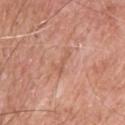The lesion was tiled from a total-body skin photograph and was not biopsied.
The tile uses white-light illumination.
A male patient, approximately 60 years of age.
About 3 mm across.
The lesion is located on the upper back.
A 15 mm close-up tile from a total-body photography series done for melanoma screening.
Automated tile analysis of the lesion measured a footprint of about 2.5 mm², a shape eccentricity near 0.95, and a shape-asymmetry score of about 0.4 (0 = symmetric). The software also gave a nevus-likeness score of about 0/100 and a lesion-detection confidence of about 95/100.